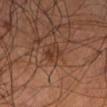<case>
  <biopsy_status>not biopsied; imaged during a skin examination</biopsy_status>
  <patient>
    <sex>male</sex>
    <age_approx>55</age_approx>
  </patient>
  <site>left lower leg</site>
  <automated_metrics>
    <area_mm2_approx>4.0</area_mm2_approx>
    <eccentricity>0.8</eccentricity>
    <shape_asymmetry>0.25</shape_asymmetry>
    <cielab_L>32</cielab_L>
    <cielab_a>20</cielab_a>
    <cielab_b>26</cielab_b>
    <vs_skin_darker_L>6.0</vs_skin_darker_L>
    <vs_skin_contrast_norm>6.5</vs_skin_contrast_norm>
  </automated_metrics>
  <image>
    <source>total-body photography crop</source>
    <field_of_view_mm>15</field_of_view_mm>
  </image>
</case>